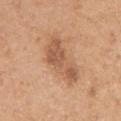Q: Was this lesion biopsied?
A: imaged on a skin check; not biopsied
Q: What is the anatomic site?
A: the left upper arm
Q: How was this image acquired?
A: total-body-photography crop, ~15 mm field of view
Q: Who is the patient?
A: female, approximately 40 years of age
Q: What lighting was used for the tile?
A: white-light
Q: What did automated image analysis measure?
A: an area of roughly 13 mm²; an average lesion color of about L≈57 a*≈22 b*≈34 (CIELAB), a lesion–skin lightness drop of about 11, and a lesion-to-skin contrast of about 7 (normalized; higher = more distinct); a border-irregularity index near 4.5/10 and internal color variation of about 4 on a 0–10 scale; a detector confidence of about 100 out of 100 that the crop contains a lesion
Q: Lesion size?
A: ≈7 mm